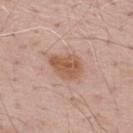Notes:
* follow-up: no biopsy performed (imaged during a skin exam)
* location: the upper back
* diameter: ≈4 mm
* acquisition: ~15 mm tile from a whole-body skin photo
* subject: male, aged around 70
* illumination: white-light illumination